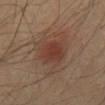Captured during whole-body skin photography for melanoma surveillance; the lesion was not biopsied.
The lesion is located on the mid back.
A male subject aged 63–67.
An algorithmic analysis of the crop reported a footprint of about 24 mm², an outline eccentricity of about 0.75 (0 = round, 1 = elongated), and a symmetry-axis asymmetry near 0.35. It also reported a mean CIELAB color near L≈40 a*≈18 b*≈25, a lesion–skin lightness drop of about 7, and a normalized border contrast of about 6. It also reported an automated nevus-likeness rating near 85 out of 100 and a lesion-detection confidence of about 100/100.
The tile uses cross-polarized illumination.
Longest diameter approximately 7 mm.
Cropped from a whole-body photographic skin survey; the tile spans about 15 mm.